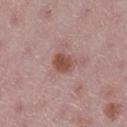Part of a total-body skin-imaging series; this lesion was reviewed on a skin check and was not flagged for biopsy.
The lesion is on the leg.
A 15 mm crop from a total-body photograph taken for skin-cancer surveillance.
A female patient roughly 45 years of age.